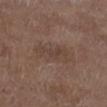Imaged during a routine full-body skin examination; the lesion was not biopsied and no histopathology is available. Imaged with white-light lighting. On the leg. A male subject, aged 73 to 77. The recorded lesion diameter is about 5.5 mm. Automated image analysis of the tile measured an area of roughly 12 mm², a shape eccentricity near 0.85, and a shape-asymmetry score of about 0.2 (0 = symmetric). And it measured a lesion color around L≈42 a*≈15 b*≈23 in CIELAB, roughly 5 lightness units darker than nearby skin, and a normalized border contrast of about 5. The analysis additionally found a classifier nevus-likeness of about 0/100 and a detector confidence of about 100 out of 100 that the crop contains a lesion. A roughly 15 mm field-of-view crop from a total-body skin photograph.